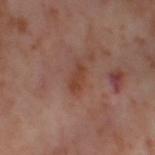{"biopsy_status": "not biopsied; imaged during a skin examination", "automated_metrics": {"cielab_L": 42, "cielab_a": 22, "cielab_b": 28, "vs_skin_darker_L": 7.0, "vs_skin_contrast_norm": 7.0, "border_irregularity_0_10": 4.0, "peripheral_color_asymmetry": 0.5, "nevus_likeness_0_100": 0, "lesion_detection_confidence_0_100": 100}, "image": {"source": "total-body photography crop", "field_of_view_mm": 15}, "patient": {"sex": "female", "age_approx": 55}, "site": "left thigh", "lighting": "cross-polarized"}